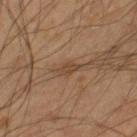Findings:
• workup · catalogued during a skin exam; not biopsied
• image-analysis metrics · a border-irregularity rating of about 4/10, internal color variation of about 1 on a 0–10 scale, and radial color variation of about 0.5; an automated nevus-likeness rating near 0 out of 100 and a detector confidence of about 95 out of 100 that the crop contains a lesion
• size · about 2.5 mm
• acquisition · 15 mm crop, total-body photography
• lighting · cross-polarized
• subject · male, aged 38–42
• anatomic site · the right lower leg